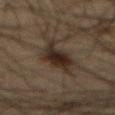{"biopsy_status": "not biopsied; imaged during a skin examination", "lesion_size": {"long_diameter_mm_approx": 6.5}, "patient": {"sex": "male", "age_approx": 65}, "lighting": "cross-polarized", "site": "abdomen", "image": {"source": "total-body photography crop", "field_of_view_mm": 15}}